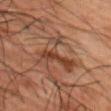* biopsy status — total-body-photography surveillance lesion; no biopsy
* patient — male, about 50 years old
* automated metrics — a border-irregularity rating of about 9/10, internal color variation of about 5.5 on a 0–10 scale, and peripheral color asymmetry of about 1.5; a nevus-likeness score of about 0/100 and a lesion-detection confidence of about 85/100
* image — 15 mm crop, total-body photography
* site — the chest
* lighting — cross-polarized
* diameter — about 5.5 mm